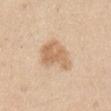– biopsy status — imaged on a skin check; not biopsied
– illumination — white-light illumination
– imaging modality — 15 mm crop, total-body photography
– anatomic site — the right upper arm
– lesion size — ≈5 mm
– subject — male, aged around 45